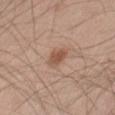The lesion was tiled from a total-body skin photograph and was not biopsied.
A close-up tile cropped from a whole-body skin photograph, about 15 mm across.
The tile uses white-light illumination.
The lesion's longest dimension is about 3 mm.
The patient is a male about 60 years old.
The lesion is located on the right thigh.